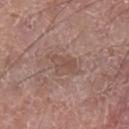notes: catalogued during a skin exam; not biopsied
lesion size: about 4 mm
location: the left lower leg
lighting: white-light
patient: male, about 60 years old
image: 15 mm crop, total-body photography